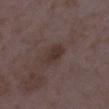Recorded during total-body skin imaging; not selected for excision or biopsy.
A female subject, approximately 35 years of age.
Approximately 2.5 mm at its widest.
Imaged with white-light lighting.
Located on the right lower leg.
This image is a 15 mm lesion crop taken from a total-body photograph.